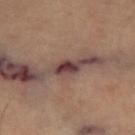A roughly 15 mm field-of-view crop from a total-body skin photograph. On the left thigh. A female subject aged 68–72.